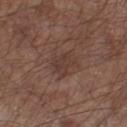Acquisition and patient details: A 15 mm crop from a total-body photograph taken for skin-cancer surveillance. On the right lower leg. Longest diameter approximately 3 mm. Captured under white-light illumination. A male subject aged around 55. The total-body-photography lesion software estimated an area of roughly 6 mm², an outline eccentricity of about 0.6 (0 = round, 1 = elongated), and a symmetry-axis asymmetry near 0.4. And it measured a lesion color around L≈37 a*≈18 b*≈23 in CIELAB and a normalized border contrast of about 5.5. It also reported a detector confidence of about 100 out of 100 that the crop contains a lesion.